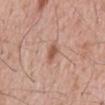Notes:
• follow-up — imaged on a skin check; not biopsied
• body site — the abdomen
• image source — ~15 mm crop, total-body skin-cancer survey
• subject — male, approximately 60 years of age